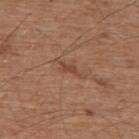Captured during whole-body skin photography for melanoma surveillance; the lesion was not biopsied.
An algorithmic analysis of the crop reported a mean CIELAB color near L≈45 a*≈22 b*≈30 and a lesion-to-skin contrast of about 6 (normalized; higher = more distinct). And it measured a border-irregularity index near 5/10 and peripheral color asymmetry of about 0.
Measured at roughly 3 mm in maximum diameter.
The lesion is on the upper back.
A lesion tile, about 15 mm wide, cut from a 3D total-body photograph.
A male subject aged 73 to 77.
Imaged with white-light lighting.